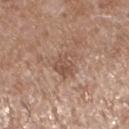subject: male, aged approximately 75
lesion size: ~3 mm (longest diameter)
TBP lesion metrics: an eccentricity of roughly 0.6 and a symmetry-axis asymmetry near 0.4; a classifier nevus-likeness of about 0/100 and lesion-presence confidence of about 100/100
body site: the left lower leg
imaging modality: 15 mm crop, total-body photography